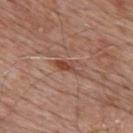Notes:
– follow-up — no biopsy performed (imaged during a skin exam)
– tile lighting — white-light
– location — the upper back
– lesion size — ≈3 mm
– TBP lesion metrics — a lesion color around L≈46 a*≈24 b*≈29 in CIELAB, roughly 10 lightness units darker than nearby skin, and a normalized lesion–skin contrast near 8; a border-irregularity rating of about 4/10, a color-variation rating of about 6/10, and peripheral color asymmetry of about 2; a nevus-likeness score of about 75/100 and a detector confidence of about 100 out of 100 that the crop contains a lesion
– patient — male, aged 78 to 82
– image — 15 mm crop, total-body photography